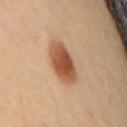Impression:
No biopsy was performed on this lesion — it was imaged during a full skin examination and was not determined to be concerning.
Context:
A roughly 15 mm field-of-view crop from a total-body skin photograph. Longest diameter approximately 5 mm. Automated tile analysis of the lesion measured a mean CIELAB color near L≈52 a*≈23 b*≈35 and a normalized border contrast of about 10.5. It also reported a border-irregularity index near 1.5/10 and peripheral color asymmetry of about 1. The analysis additionally found an automated nevus-likeness rating near 100 out of 100 and a detector confidence of about 100 out of 100 that the crop contains a lesion. The patient is a female aged 33 to 37. On the upper back. Imaged with cross-polarized lighting.